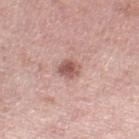image-analysis metrics: a footprint of about 4.5 mm², a shape eccentricity near 0.5, and a shape-asymmetry score of about 0.25 (0 = symmetric); a lesion color around L≈56 a*≈22 b*≈24 in CIELAB, a lesion–skin lightness drop of about 13, and a normalized lesion–skin contrast near 8; a border-irregularity rating of about 2.5/10 and radial color variation of about 1 | subject: female, aged 38 to 42 | acquisition: total-body-photography crop, ~15 mm field of view | anatomic site: the leg | illumination: white-light illumination | lesion size: ~2.5 mm (longest diameter).